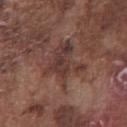Captured during whole-body skin photography for melanoma surveillance; the lesion was not biopsied.
On the chest.
This is a white-light tile.
The recorded lesion diameter is about 5.5 mm.
A roughly 15 mm field-of-view crop from a total-body skin photograph.
A male subject approximately 75 years of age.